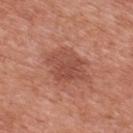Case summary:
• notes — total-body-photography surveillance lesion; no biopsy